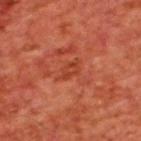Q: Was a biopsy performed?
A: catalogued during a skin exam; not biopsied
Q: What lighting was used for the tile?
A: cross-polarized
Q: Who is the patient?
A: male, about 65 years old
Q: What kind of image is this?
A: ~15 mm crop, total-body skin-cancer survey
Q: Lesion location?
A: the upper back
Q: Lesion size?
A: about 3 mm